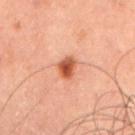The lesion was tiled from a total-body skin photograph and was not biopsied. Imaged with cross-polarized lighting. On the mid back. A close-up tile cropped from a whole-body skin photograph, about 15 mm across. The patient is a male aged 58–62. About 2.5 mm across.